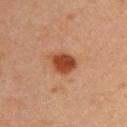Captured during whole-body skin photography for melanoma surveillance; the lesion was not biopsied. The recorded lesion diameter is about 3.5 mm. The patient is a female roughly 35 years of age. The lesion-visualizer software estimated a footprint of about 7 mm². And it measured an average lesion color of about L≈46 a*≈29 b*≈36 (CIELAB), about 14 CIELAB-L* units darker than the surrounding skin, and a normalized border contrast of about 11. The software also gave a border-irregularity rating of about 2/10, a color-variation rating of about 3/10, and a peripheral color-asymmetry measure near 1. On the upper back. The tile uses cross-polarized illumination. A 15 mm crop from a total-body photograph taken for skin-cancer surveillance.